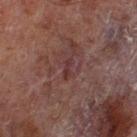Captured during whole-body skin photography for melanoma surveillance; the lesion was not biopsied.
This is a cross-polarized tile.
The total-body-photography lesion software estimated an area of roughly 2 mm², an outline eccentricity of about 0.9 (0 = round, 1 = elongated), and two-axis asymmetry of about 0.4. The software also gave a lesion color around L≈26 a*≈18 b*≈16 in CIELAB, about 6 CIELAB-L* units darker than the surrounding skin, and a lesion-to-skin contrast of about 6.5 (normalized; higher = more distinct). The analysis additionally found border irregularity of about 4.5 on a 0–10 scale, internal color variation of about 0 on a 0–10 scale, and peripheral color asymmetry of about 0. The analysis additionally found a classifier nevus-likeness of about 0/100.
A male subject, aged 68–72.
The lesion is located on the left thigh.
A 15 mm close-up tile from a total-body photography series done for melanoma screening.